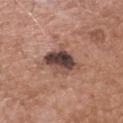| key | value |
|---|---|
| workup | imaged on a skin check; not biopsied |
| diameter | ≈4.5 mm |
| TBP lesion metrics | a footprint of about 12 mm² and two-axis asymmetry of about 0.3 |
| subject | male, aged around 50 |
| acquisition | total-body-photography crop, ~15 mm field of view |
| tile lighting | white-light |
| location | the head or neck |